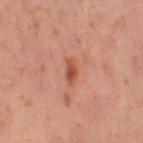The lesion was photographed on a routine skin check and not biopsied; there is no pathology result.
The lesion-visualizer software estimated a symmetry-axis asymmetry near 0.25. It also reported a mean CIELAB color near L≈52 a*≈28 b*≈33, roughly 10 lightness units darker than nearby skin, and a normalized border contrast of about 7.5. And it measured a border-irregularity rating of about 2.5/10 and a within-lesion color-variation index near 3/10. The analysis additionally found an automated nevus-likeness rating near 55 out of 100.
From the left thigh.
The patient is a female aged approximately 40.
A 15 mm close-up tile from a total-body photography series done for melanoma screening.
The recorded lesion diameter is about 3.5 mm.
Imaged with cross-polarized lighting.